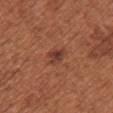Part of a total-body skin-imaging series; this lesion was reviewed on a skin check and was not flagged for biopsy. The lesion is on the chest. This is a white-light tile. Cropped from a whole-body photographic skin survey; the tile spans about 15 mm. A female subject roughly 65 years of age. The total-body-photography lesion software estimated an area of roughly 4 mm² and a shape-asymmetry score of about 0.35 (0 = symmetric). The software also gave a lesion–skin lightness drop of about 9 and a lesion-to-skin contrast of about 8 (normalized; higher = more distinct). It also reported a classifier nevus-likeness of about 85/100 and a lesion-detection confidence of about 100/100.